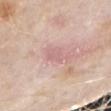A close-up tile cropped from a whole-body skin photograph, about 15 mm across. The subject is a male in their mid- to late 70s. On the right upper arm. Measured at roughly 3.5 mm in maximum diameter. Captured under white-light illumination.